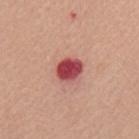Notes:
- tile lighting · white-light
- subject · male, aged 63 to 67
- body site · the mid back
- image · ~15 mm tile from a whole-body skin photo
- lesion diameter · ~3 mm (longest diameter)